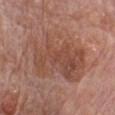Findings:
– follow-up · no biopsy performed (imaged during a skin exam)
– subject · male, aged approximately 80
– illumination · white-light illumination
– TBP lesion metrics · roughly 7 lightness units darker than nearby skin and a normalized lesion–skin contrast near 6; a border-irregularity rating of about 5/10, a color-variation rating of about 4.5/10, and a peripheral color-asymmetry measure near 1.5; a detector confidence of about 100 out of 100 that the crop contains a lesion
– size · ~8.5 mm (longest diameter)
– image · 15 mm crop, total-body photography
– body site · the front of the torso